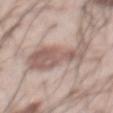Part of a total-body skin-imaging series; this lesion was reviewed on a skin check and was not flagged for biopsy.
The lesion-visualizer software estimated border irregularity of about 6 on a 0–10 scale, internal color variation of about 3.5 on a 0–10 scale, and radial color variation of about 1.5.
A 15 mm crop from a total-body photograph taken for skin-cancer surveillance.
The subject is a male approximately 55 years of age.
Measured at roughly 6 mm in maximum diameter.
Imaged with white-light lighting.
From the abdomen.